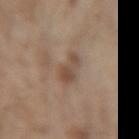Assessment: Imaged during a routine full-body skin examination; the lesion was not biopsied and no histopathology is available. Acquisition and patient details: Imaged with white-light lighting. An algorithmic analysis of the crop reported a lesion area of about 6.5 mm², an outline eccentricity of about 0.85 (0 = round, 1 = elongated), and a shape-asymmetry score of about 0.25 (0 = symmetric). And it measured border irregularity of about 3 on a 0–10 scale, internal color variation of about 4.5 on a 0–10 scale, and peripheral color asymmetry of about 1.5. And it measured a nevus-likeness score of about 5/100. A male subject, about 70 years old. The lesion is on the left forearm. Cropped from a whole-body photographic skin survey; the tile spans about 15 mm. Longest diameter approximately 3.5 mm.